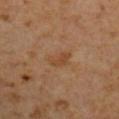<lesion>
  <biopsy_status>not biopsied; imaged during a skin examination</biopsy_status>
  <image>
    <source>total-body photography crop</source>
    <field_of_view_mm>15</field_of_view_mm>
  </image>
  <site>right upper arm</site>
  <patient>
    <sex>male</sex>
    <age_approx>60</age_approx>
  </patient>
  <lesion_size>
    <long_diameter_mm_approx>3.0</long_diameter_mm_approx>
  </lesion_size>
  <lighting>cross-polarized</lighting>
  <automated_metrics>
    <area_mm2_approx>4.0</area_mm2_approx>
    <shape_asymmetry>0.35</shape_asymmetry>
    <border_irregularity_0_10>3.5</border_irregularity_0_10>
    <peripheral_color_asymmetry>0.5</peripheral_color_asymmetry>
  </automated_metrics>
</lesion>